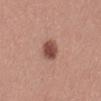biopsy status — total-body-photography surveillance lesion; no biopsy | tile lighting — white-light | patient — female, aged approximately 40 | image source — ~15 mm tile from a whole-body skin photo | body site — the left thigh.